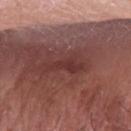location — the right forearm
subject — male, approximately 75 years of age
acquisition — ~15 mm tile from a whole-body skin photo